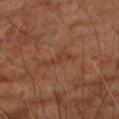Assessment: The lesion was tiled from a total-body skin photograph and was not biopsied. Acquisition and patient details: This image is a 15 mm lesion crop taken from a total-body photograph. Automated tile analysis of the lesion measured an outline eccentricity of about 0.95 (0 = round, 1 = elongated) and a shape-asymmetry score of about 0.55 (0 = symmetric). The analysis additionally found a lesion–skin lightness drop of about 5 and a normalized lesion–skin contrast near 4.5. The software also gave a border-irregularity rating of about 6/10 and radial color variation of about 0. A male subject roughly 65 years of age. On the right upper arm.